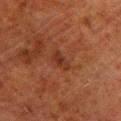Clinical impression:
The lesion was tiled from a total-body skin photograph and was not biopsied.
Image and clinical context:
This image is a 15 mm lesion crop taken from a total-body photograph. A male subject about 80 years old. On the left lower leg.